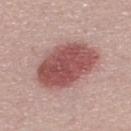This image is a 15 mm lesion crop taken from a total-body photograph. A male patient, aged approximately 30. The tile uses white-light illumination.